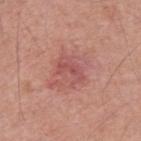Impression:
Captured during whole-body skin photography for melanoma surveillance; the lesion was not biopsied.
Clinical summary:
Imaged with white-light lighting. Automated image analysis of the tile measured a lesion color around L≈53 a*≈28 b*≈24 in CIELAB and about 8 CIELAB-L* units darker than the surrounding skin. The analysis additionally found border irregularity of about 5 on a 0–10 scale, a within-lesion color-variation index near 2.5/10, and a peripheral color-asymmetry measure near 1. The software also gave a classifier nevus-likeness of about 0/100 and lesion-presence confidence of about 100/100. On the upper back. A male patient, approximately 50 years of age. A 15 mm close-up extracted from a 3D total-body photography capture.